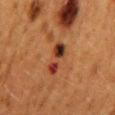Findings:
* notes — total-body-photography surveillance lesion; no biopsy
* imaging modality — total-body-photography crop, ~15 mm field of view
* TBP lesion metrics — a classifier nevus-likeness of about 0/100 and a lesion-detection confidence of about 100/100
* subject — female, in their 50s
* size — ~4.5 mm (longest diameter)
* body site — the mid back
* illumination — cross-polarized illumination